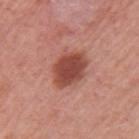follow-up — catalogued during a skin exam; not biopsied
diameter — ≈4.5 mm
subject — female, aged 53–57
tile lighting — white-light
image — total-body-photography crop, ~15 mm field of view
location — the left upper arm
TBP lesion metrics — a lesion area of about 12 mm², an eccentricity of roughly 0.55, and two-axis asymmetry of about 0.15; a lesion color around L≈47 a*≈28 b*≈28 in CIELAB and roughly 14 lightness units darker than nearby skin